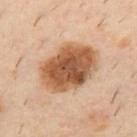workup: total-body-photography surveillance lesion; no biopsy
anatomic site: the upper back
patient: male, roughly 40 years of age
imaging modality: ~15 mm tile from a whole-body skin photo